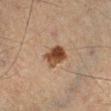Imaged during a routine full-body skin examination; the lesion was not biopsied and no histopathology is available. About 3 mm across. Cropped from a total-body skin-imaging series; the visible field is about 15 mm. Imaged with cross-polarized lighting. On the leg. The total-body-photography lesion software estimated an average lesion color of about L≈37 a*≈17 b*≈27 (CIELAB), a lesion–skin lightness drop of about 13, and a normalized border contrast of about 11. It also reported border irregularity of about 2 on a 0–10 scale, a within-lesion color-variation index near 6.5/10, and peripheral color asymmetry of about 2.5. The subject is a male aged around 60.